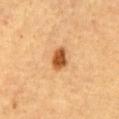The lesion was tiled from a total-body skin photograph and was not biopsied.